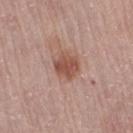Recorded during total-body skin imaging; not selected for excision or biopsy. A 15 mm close-up extracted from a 3D total-body photography capture. A female subject, in their mid-60s. On the leg.